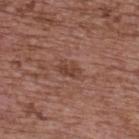Q: Was a biopsy performed?
A: no biopsy performed (imaged during a skin exam)
Q: What lighting was used for the tile?
A: white-light illumination
Q: Automated lesion metrics?
A: a mean CIELAB color near L≈42 a*≈21 b*≈26; a classifier nevus-likeness of about 0/100 and lesion-presence confidence of about 100/100
Q: How was this image acquired?
A: 15 mm crop, total-body photography
Q: Lesion size?
A: about 2.5 mm
Q: What are the patient's age and sex?
A: female, aged around 65
Q: Where on the body is the lesion?
A: the back